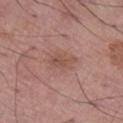  biopsy_status: not biopsied; imaged during a skin examination
  image:
    source: total-body photography crop
    field_of_view_mm: 15
  patient:
    sex: male
    age_approx: 70
  site: right lower leg
  automated_metrics:
    cielab_L: 51
    cielab_a: 22
    cielab_b: 25
    vs_skin_contrast_norm: 5.5
  lighting: white-light
  lesion_size:
    long_diameter_mm_approx: 3.5